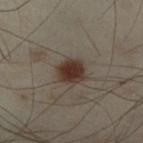lesion size: ~4 mm (longest diameter)
acquisition: ~15 mm tile from a whole-body skin photo
anatomic site: the left leg
TBP lesion metrics: a footprint of about 8.5 mm², an outline eccentricity of about 0.6 (0 = round, 1 = elongated), and a symmetry-axis asymmetry near 0.15; a mean CIELAB color near L≈26 a*≈11 b*≈18 and about 10 CIELAB-L* units darker than the surrounding skin; border irregularity of about 1.5 on a 0–10 scale, a within-lesion color-variation index near 3/10, and a peripheral color-asymmetry measure near 1; an automated nevus-likeness rating near 100 out of 100 and a lesion-detection confidence of about 100/100
lighting: cross-polarized
subject: male, aged approximately 50